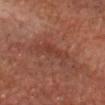Clinical impression:
The lesion was tiled from a total-body skin photograph and was not biopsied.
Background:
A male patient, aged 58–62. A close-up tile cropped from a whole-body skin photograph, about 15 mm across. The lesion is located on the head or neck.